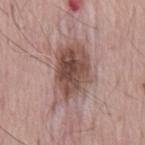biopsy_status: not biopsied; imaged during a skin examination
lighting: white-light
automated_metrics:
  eccentricity: 0.8
  shape_asymmetry: 0.25
  cielab_L: 49
  cielab_a: 19
  cielab_b: 22
  vs_skin_darker_L: 14.0
  vs_skin_contrast_norm: 9.5
  border_irregularity_0_10: 3.5
  nevus_likeness_0_100: 80
image:
  source: total-body photography crop
  field_of_view_mm: 15
patient:
  sex: male
  age_approx: 55
lesion_size:
  long_diameter_mm_approx: 7.0